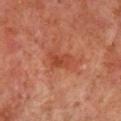Part of a total-body skin-imaging series; this lesion was reviewed on a skin check and was not flagged for biopsy.
Imaged with cross-polarized lighting.
A 15 mm crop from a total-body photograph taken for skin-cancer surveillance.
A male subject, about 65 years old.
The total-body-photography lesion software estimated an outline eccentricity of about 0.75 (0 = round, 1 = elongated). It also reported about 7 CIELAB-L* units darker than the surrounding skin. The analysis additionally found border irregularity of about 3 on a 0–10 scale, internal color variation of about 2.5 on a 0–10 scale, and peripheral color asymmetry of about 1. And it measured an automated nevus-likeness rating near 0 out of 100 and a lesion-detection confidence of about 100/100.
The lesion is located on the chest.